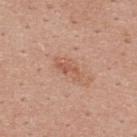Captured during whole-body skin photography for melanoma surveillance; the lesion was not biopsied.
A lesion tile, about 15 mm wide, cut from a 3D total-body photograph.
Located on the upper back.
A male subject in their mid- to late 20s.